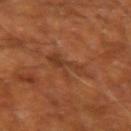| feature | finding |
|---|---|
| follow-up | no biopsy performed (imaged during a skin exam) |
| acquisition | ~15 mm crop, total-body skin-cancer survey |
| subject | male, roughly 65 years of age |
| body site | the left upper arm |